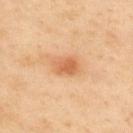Assessment: The lesion was tiled from a total-body skin photograph and was not biopsied. Background: The subject is a male aged 53 to 57. Located on the upper back. Imaged with cross-polarized lighting. Longest diameter approximately 3 mm. A roughly 15 mm field-of-view crop from a total-body skin photograph.